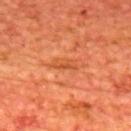{"biopsy_status": "not biopsied; imaged during a skin examination"}